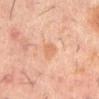Part of a total-body skin-imaging series; this lesion was reviewed on a skin check and was not flagged for biopsy.
The subject is a male aged around 60.
Captured under cross-polarized illumination.
From the mid back.
A region of skin cropped from a whole-body photographic capture, roughly 15 mm wide.